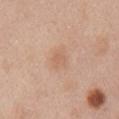follow-up: total-body-photography surveillance lesion; no biopsy
subject: female, approximately 50 years of age
size: ≈2.5 mm
image-analysis metrics: an area of roughly 4.5 mm² and a shape-asymmetry score of about 0.15 (0 = symmetric); a lesion color around L≈63 a*≈19 b*≈32 in CIELAB, roughly 6 lightness units darker than nearby skin, and a lesion-to-skin contrast of about 4.5 (normalized; higher = more distinct); a peripheral color-asymmetry measure near 0.5
acquisition: total-body-photography crop, ~15 mm field of view
illumination: white-light illumination
site: the right upper arm